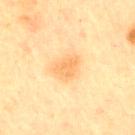Assessment:
The lesion was photographed on a routine skin check and not biopsied; there is no pathology result.
Background:
A region of skin cropped from a whole-body photographic capture, roughly 15 mm wide. The tile uses cross-polarized illumination. An algorithmic analysis of the crop reported a within-lesion color-variation index near 2/10 and peripheral color asymmetry of about 0.5. The software also gave an automated nevus-likeness rating near 45 out of 100 and lesion-presence confidence of about 100/100. The subject is a male aged 63–67. Measured at roughly 3.5 mm in maximum diameter. From the arm.